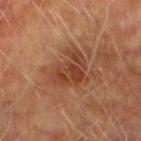This lesion was catalogued during total-body skin photography and was not selected for biopsy. The recorded lesion diameter is about 5 mm. Imaged with cross-polarized lighting. A 15 mm close-up tile from a total-body photography series done for melanoma screening. The patient is a male in their mid- to late 70s. The lesion is located on the left lower leg.